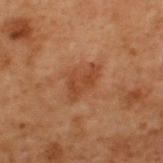Clinical impression: The lesion was tiled from a total-body skin photograph and was not biopsied. Image and clinical context: Imaged with cross-polarized lighting. Cropped from a whole-body photographic skin survey; the tile spans about 15 mm. The lesion is on the upper back. A male patient, aged 68 to 72.